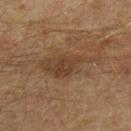{"biopsy_status": "not biopsied; imaged during a skin examination", "patient": {"sex": "male", "age_approx": 50}, "site": "left forearm", "image": {"source": "total-body photography crop", "field_of_view_mm": 15}}